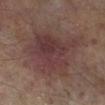<lesion>
  <biopsy_status>not biopsied; imaged during a skin examination</biopsy_status>
  <automated_metrics>
    <area_mm2_approx>32.0</area_mm2_approx>
    <eccentricity>0.7</eccentricity>
    <shape_asymmetry>0.4</shape_asymmetry>
    <cielab_L>35</cielab_L>
    <cielab_a>18</cielab_a>
    <cielab_b>17</cielab_b>
    <vs_skin_contrast_norm>7.5</vs_skin_contrast_norm>
    <border_irregularity_0_10>5.5</border_irregularity_0_10>
    <peripheral_color_asymmetry>1.5</peripheral_color_asymmetry>
  </automated_metrics>
  <lighting>cross-polarized</lighting>
  <lesion_size>
    <long_diameter_mm_approx>8.5</long_diameter_mm_approx>
  </lesion_size>
  <patient>
    <sex>male</sex>
    <age_approx>65</age_approx>
  </patient>
  <image>
    <source>total-body photography crop</source>
    <field_of_view_mm>15</field_of_view_mm>
  </image>
  <site>right lower leg</site>
</lesion>